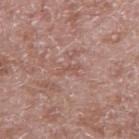<tbp_lesion>
<lesion_size>
  <long_diameter_mm_approx>2.5</long_diameter_mm_approx>
</lesion_size>
<patient>
  <sex>male</sex>
  <age_approx>50</age_approx>
</patient>
<lighting>white-light</lighting>
<site>right upper arm</site>
<automated_metrics>
  <area_mm2_approx>2.5</area_mm2_approx>
  <eccentricity>0.9</eccentricity>
  <border_irregularity_0_10>5.5</border_irregularity_0_10>
  <color_variation_0_10>0.0</color_variation_0_10>
  <peripheral_color_asymmetry>0.0</peripheral_color_asymmetry>
  <nevus_likeness_0_100>0</nevus_likeness_0_100>
  <lesion_detection_confidence_0_100>75</lesion_detection_confidence_0_100>
</automated_metrics>
<image>
  <source>total-body photography crop</source>
  <field_of_view_mm>15</field_of_view_mm>
</image>
</tbp_lesion>